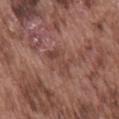| key | value |
|---|---|
| notes | no biopsy performed (imaged during a skin exam) |
| imaging modality | ~15 mm crop, total-body skin-cancer survey |
| subject | male, aged 73–77 |
| automated metrics | a lesion area of about 6 mm², a shape eccentricity near 0.75, and two-axis asymmetry of about 0.4; a mean CIELAB color near L≈44 a*≈21 b*≈24, a lesion–skin lightness drop of about 7, and a normalized lesion–skin contrast near 5.5 |
| illumination | white-light |
| lesion diameter | ~3.5 mm (longest diameter) |
| site | the back |